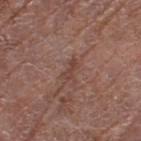Recorded during total-body skin imaging; not selected for excision or biopsy.
A 15 mm close-up extracted from a 3D total-body photography capture.
A female patient, aged 78–82.
Located on the left thigh.